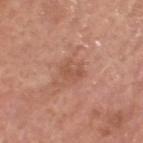size = ~3 mm (longest diameter)
body site = the head or neck
acquisition = ~15 mm crop, total-body skin-cancer survey
image-analysis metrics = an automated nevus-likeness rating near 0 out of 100 and a lesion-detection confidence of about 100/100
patient = male, aged approximately 65